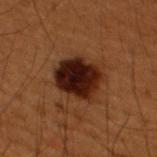Assessment: This lesion was catalogued during total-body skin photography and was not selected for biopsy. Background: The subject is a male aged approximately 50. Located on the upper back. The recorded lesion diameter is about 5 mm. This image is a 15 mm lesion crop taken from a total-body photograph. This is a cross-polarized tile. Automated image analysis of the tile measured a border-irregularity rating of about 1.5/10, internal color variation of about 7 on a 0–10 scale, and peripheral color asymmetry of about 2. The analysis additionally found an automated nevus-likeness rating near 95 out of 100.